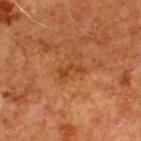workup — total-body-photography surveillance lesion; no biopsy | anatomic site — the upper back | patient — male, aged 78–82 | imaging modality — ~15 mm tile from a whole-body skin photo | tile lighting — cross-polarized illumination.